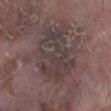Q: Was this lesion biopsied?
A: total-body-photography surveillance lesion; no biopsy
Q: What lighting was used for the tile?
A: white-light
Q: Who is the patient?
A: male, aged 73 to 77
Q: What is the imaging modality?
A: total-body-photography crop, ~15 mm field of view
Q: Lesion location?
A: the leg